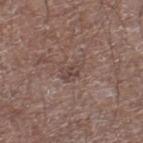workup=catalogued during a skin exam; not biopsied
image source=total-body-photography crop, ~15 mm field of view
size=~3 mm (longest diameter)
location=the leg
TBP lesion metrics=a footprint of about 4.5 mm², a shape eccentricity near 0.8, and two-axis asymmetry of about 0.35; a mean CIELAB color near L≈44 a*≈16 b*≈21 and a normalized lesion–skin contrast near 6; a nevus-likeness score of about 0/100 and a detector confidence of about 90 out of 100 that the crop contains a lesion
lighting=white-light
subject=male, in their mid- to late 60s